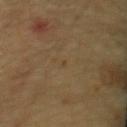* notes — total-body-photography surveillance lesion; no biopsy
* image — total-body-photography crop, ~15 mm field of view
* size — about 1 mm
* patient — female, approximately 50 years of age
* location — the mid back